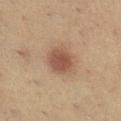workup = imaged on a skin check; not biopsied | size = ≈3.5 mm | anatomic site = the right lower leg | automated lesion analysis = a lesion area of about 8.5 mm², a shape eccentricity near 0.55, and a symmetry-axis asymmetry near 0.15; a border-irregularity rating of about 1/10, a color-variation rating of about 2.5/10, and radial color variation of about 1; a nevus-likeness score of about 100/100 and a detector confidence of about 100 out of 100 that the crop contains a lesion | illumination = cross-polarized | patient = female, about 55 years old | image = ~15 mm tile from a whole-body skin photo.